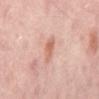follow-up: total-body-photography surveillance lesion; no biopsy
lesion size: about 3 mm
image: ~15 mm crop, total-body skin-cancer survey
location: the mid back
automated metrics: a footprint of about 4.5 mm² and two-axis asymmetry of about 0.2; a mean CIELAB color near L≈63 a*≈23 b*≈29 and about 9 CIELAB-L* units darker than the surrounding skin; a border-irregularity rating of about 2/10, a color-variation rating of about 3/10, and radial color variation of about 1
patient: aged 53–57